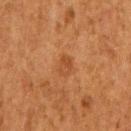Image and clinical context:
The patient is a female aged around 50. A 15 mm close-up extracted from a 3D total-body photography capture. The lesion is located on the right upper arm. The total-body-photography lesion software estimated a shape-asymmetry score of about 0.35 (0 = symmetric). The software also gave border irregularity of about 3 on a 0–10 scale, a color-variation rating of about 2.5/10, and peripheral color asymmetry of about 1. It also reported an automated nevus-likeness rating near 35 out of 100. This is a cross-polarized tile.